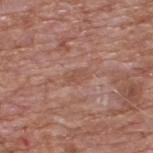No biopsy was performed on this lesion — it was imaged during a full skin examination and was not determined to be concerning.
Automated tile analysis of the lesion measured a lesion area of about 3 mm² and an outline eccentricity of about 0.85 (0 = round, 1 = elongated). And it measured an average lesion color of about L≈51 a*≈22 b*≈28 (CIELAB), a lesion–skin lightness drop of about 6, and a lesion-to-skin contrast of about 5 (normalized; higher = more distinct). It also reported a border-irregularity rating of about 2.5/10, a within-lesion color-variation index near 0.5/10, and peripheral color asymmetry of about 0. And it measured a classifier nevus-likeness of about 0/100 and a detector confidence of about 55 out of 100 that the crop contains a lesion.
From the back.
This image is a 15 mm lesion crop taken from a total-body photograph.
A male patient, approximately 60 years of age.
The lesion's longest dimension is about 2.5 mm.